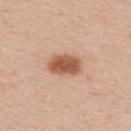image=~15 mm crop, total-body skin-cancer survey | tile lighting=white-light | anatomic site=the back | patient=female, aged 33 to 37.